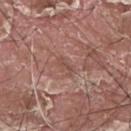follow-up: catalogued during a skin exam; not biopsied | automated metrics: a lesion area of about 2.5 mm², a shape eccentricity near 0.9, and a shape-asymmetry score of about 0.4 (0 = symmetric); an average lesion color of about L≈49 a*≈21 b*≈25 (CIELAB), a lesion–skin lightness drop of about 7, and a normalized border contrast of about 5.5; a border-irregularity rating of about 5/10 and a color-variation rating of about 0/10 | lesion size: ≈2.5 mm | image source: ~15 mm tile from a whole-body skin photo | tile lighting: white-light | body site: the upper back | subject: male, about 40 years old.